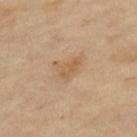Case summary:
- workup: imaged on a skin check; not biopsied
- automated lesion analysis: an average lesion color of about L≈59 a*≈19 b*≈35 (CIELAB), roughly 7 lightness units darker than nearby skin, and a lesion-to-skin contrast of about 5.5 (normalized; higher = more distinct); a nevus-likeness score of about 10/100
- body site: the right thigh
- illumination: cross-polarized illumination
- acquisition: 15 mm crop, total-body photography
- subject: female, aged 63 to 67
- diameter: about 3 mm